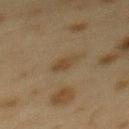biopsy status = no biopsy performed (imaged during a skin exam) | site = the mid back | patient = female, approximately 40 years of age | imaging modality = ~15 mm crop, total-body skin-cancer survey.